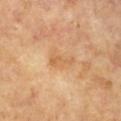workup — imaged on a skin check; not biopsied
illumination — cross-polarized
subject — female, roughly 75 years of age
imaging modality — ~15 mm crop, total-body skin-cancer survey
site — the upper back